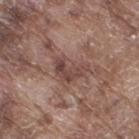This lesion was catalogued during total-body skin photography and was not selected for biopsy.
A male patient aged around 75.
Measured at roughly 4.5 mm in maximum diameter.
A region of skin cropped from a whole-body photographic capture, roughly 15 mm wide.
On the right thigh.
The tile uses white-light illumination.